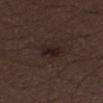Q: Automated lesion metrics?
A: a border-irregularity rating of about 3/10, internal color variation of about 2 on a 0–10 scale, and a peripheral color-asymmetry measure near 0.5
Q: Illumination type?
A: white-light illumination
Q: What kind of image is this?
A: ~15 mm tile from a whole-body skin photo
Q: Patient demographics?
A: male, aged 28 to 32
Q: What is the lesion's diameter?
A: ~3 mm (longest diameter)
Q: What is the anatomic site?
A: the left thigh